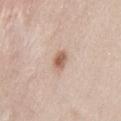{
  "biopsy_status": "not biopsied; imaged during a skin examination"
}